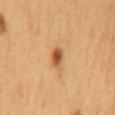Case summary:
• biopsy status · catalogued during a skin exam; not biopsied
• anatomic site · the mid back
• diameter · ~2.5 mm (longest diameter)
• subject · female, roughly 60 years of age
• automated metrics · a lesion color around L≈57 a*≈25 b*≈42 in CIELAB, a lesion–skin lightness drop of about 15, and a lesion-to-skin contrast of about 9.5 (normalized; higher = more distinct); a border-irregularity index near 1.5/10 and internal color variation of about 4 on a 0–10 scale
• image source · ~15 mm crop, total-body skin-cancer survey